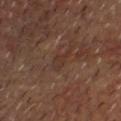Q: Was this lesion biopsied?
A: total-body-photography surveillance lesion; no biopsy
Q: What is the imaging modality?
A: 15 mm crop, total-body photography
Q: What are the patient's age and sex?
A: male, roughly 60 years of age
Q: How was the tile lit?
A: cross-polarized
Q: Where on the body is the lesion?
A: the head or neck